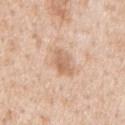Clinical impression:
The lesion was photographed on a routine skin check and not biopsied; there is no pathology result.
Image and clinical context:
An algorithmic analysis of the crop reported a border-irregularity rating of about 3/10 and internal color variation of about 2.5 on a 0–10 scale. From the mid back. Captured under white-light illumination. This image is a 15 mm lesion crop taken from a total-body photograph. A male subject approximately 65 years of age.